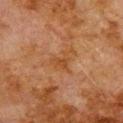Recorded during total-body skin imaging; not selected for excision or biopsy. Cropped from a whole-body photographic skin survey; the tile spans about 15 mm. The subject is a male aged 78 to 82. This is a cross-polarized tile. The lesion is on the upper back.